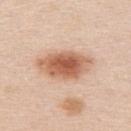workup: imaged on a skin check; not biopsied
imaging modality: 15 mm crop, total-body photography
patient: male, roughly 40 years of age
diameter: ≈6.5 mm
TBP lesion metrics: a border-irregularity index near 2/10 and internal color variation of about 6 on a 0–10 scale; a nevus-likeness score of about 100/100 and a lesion-detection confidence of about 100/100
site: the back